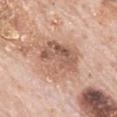Captured during whole-body skin photography for melanoma surveillance; the lesion was not biopsied. On the mid back. A male subject, aged 78 to 82. A close-up tile cropped from a whole-body skin photograph, about 15 mm across. This is a white-light tile.